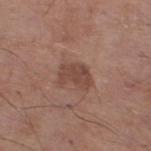biopsy status=imaged on a skin check; not biopsied
TBP lesion metrics=a lesion color around L≈45 a*≈20 b*≈25 in CIELAB and roughly 9 lightness units darker than nearby skin; border irregularity of about 3 on a 0–10 scale; lesion-presence confidence of about 100/100
site=the left lower leg
tile lighting=white-light illumination
patient=male, aged approximately 70
image=~15 mm tile from a whole-body skin photo